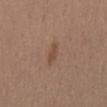This lesion was catalogued during total-body skin photography and was not selected for biopsy. About 2.5 mm across. Automated tile analysis of the lesion measured an area of roughly 2.5 mm² and a shape-asymmetry score of about 0.3 (0 = symmetric). The analysis additionally found border irregularity of about 3 on a 0–10 scale, a color-variation rating of about 0/10, and a peripheral color-asymmetry measure near 0. A female subject about 55 years old. Cropped from a whole-body photographic skin survey; the tile spans about 15 mm. Imaged with white-light lighting. Located on the mid back.